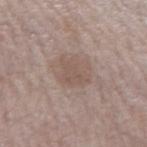{
  "biopsy_status": "not biopsied; imaged during a skin examination",
  "site": "left forearm",
  "lighting": "white-light",
  "lesion_size": {
    "long_diameter_mm_approx": 3.5
  },
  "image": {
    "source": "total-body photography crop",
    "field_of_view_mm": 15
  },
  "automated_metrics": {
    "area_mm2_approx": 6.5,
    "eccentricity": 0.7,
    "shape_asymmetry": 0.4,
    "border_irregularity_0_10": 4.5,
    "color_variation_0_10": 1.5,
    "peripheral_color_asymmetry": 0.5,
    "nevus_likeness_0_100": 35,
    "lesion_detection_confidence_0_100": 100
  },
  "patient": {
    "sex": "female",
    "age_approx": 65
  }
}